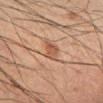workup = catalogued during a skin exam; not biopsied
image source = total-body-photography crop, ~15 mm field of view
subject = male, in their 50s
size = ~2.5 mm (longest diameter)
body site = the right lower leg
automated metrics = two-axis asymmetry of about 0.25; roughly 10 lightness units darker than nearby skin and a normalized border contrast of about 7; an automated nevus-likeness rating near 95 out of 100 and lesion-presence confidence of about 100/100
tile lighting = cross-polarized